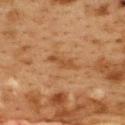biopsy_status: not biopsied; imaged during a skin examination
automated_metrics:
  area_mm2_approx: 4.5
  eccentricity: 0.95
  shape_asymmetry: 0.35
  cielab_L: 41
  cielab_a: 19
  cielab_b: 33
  vs_skin_darker_L: 7.0
  vs_skin_contrast_norm: 6.0
lighting: cross-polarized
patient:
  sex: female
  age_approx: 40
image:
  source: total-body photography crop
  field_of_view_mm: 15
lesion_size:
  long_diameter_mm_approx: 4.0
site: upper back